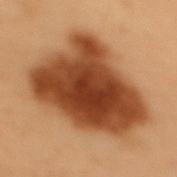workup=catalogued during a skin exam; not biopsied | lesion size=≈10.5 mm | image=~15 mm tile from a whole-body skin photo | patient=female, approximately 50 years of age | location=the upper back | lighting=cross-polarized illumination.